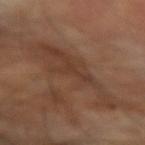Captured during whole-body skin photography for melanoma surveillance; the lesion was not biopsied. A 15 mm crop from a total-body photograph taken for skin-cancer surveillance. Captured under cross-polarized illumination. The subject is a male in their 70s. The recorded lesion diameter is about 10.5 mm. The lesion is on the left forearm.